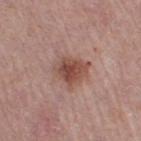Notes:
- biopsy status: total-body-photography surveillance lesion; no biopsy
- lighting: white-light
- subject: female, aged 38 to 42
- anatomic site: the right thigh
- lesion size: ~4 mm (longest diameter)
- automated lesion analysis: a footprint of about 12 mm², an outline eccentricity of about 0.45 (0 = round, 1 = elongated), and two-axis asymmetry of about 0.25; a detector confidence of about 100 out of 100 that the crop contains a lesion
- imaging modality: ~15 mm tile from a whole-body skin photo